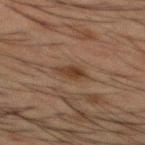Case summary:
• biopsy status — imaged on a skin check; not biopsied
• site — the back
• subject — male, aged 48 to 52
• acquisition — 15 mm crop, total-body photography